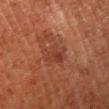{
  "image": {
    "source": "total-body photography crop",
    "field_of_view_mm": 15
  },
  "lighting": "cross-polarized",
  "patient": {
    "sex": "male",
    "age_approx": 80
  },
  "lesion_size": {
    "long_diameter_mm_approx": 3.5
  },
  "site": "left lower leg"
}